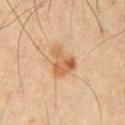Clinical impression:
The lesion was tiled from a total-body skin photograph and was not biopsied.
Clinical summary:
Located on the front of the torso. Longest diameter approximately 4 mm. A male patient roughly 40 years of age. This is a cross-polarized tile. A 15 mm close-up extracted from a 3D total-body photography capture.